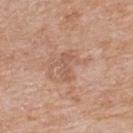Captured during whole-body skin photography for melanoma surveillance; the lesion was not biopsied.
Cropped from a total-body skin-imaging series; the visible field is about 15 mm.
A female subject, aged approximately 75.
Located on the upper back.
The lesion's longest dimension is about 3.5 mm.